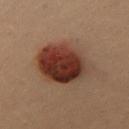Part of a total-body skin-imaging series; this lesion was reviewed on a skin check and was not flagged for biopsy. A female patient approximately 40 years of age. On the chest. Cropped from a whole-body photographic skin survey; the tile spans about 15 mm. Imaged with cross-polarized lighting.